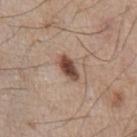| feature | finding |
|---|---|
| notes | catalogued during a skin exam; not biopsied |
| subject | male, in their mid- to late 70s |
| body site | the chest |
| imaging modality | total-body-photography crop, ~15 mm field of view |
| lesion size | ≈3.5 mm |